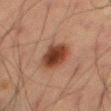Context:
The tile uses cross-polarized illumination. The total-body-photography lesion software estimated an outline eccentricity of about 0.75 (0 = round, 1 = elongated). The software also gave a classifier nevus-likeness of about 100/100. A 15 mm close-up tile from a total-body photography series done for melanoma screening. A male subject in their mid- to late 60s. About 4 mm across. From the left thigh.